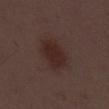The lesion was tiled from a total-body skin photograph and was not biopsied. Cropped from a whole-body photographic skin survey; the tile spans about 15 mm. A male subject, aged 48 to 52.